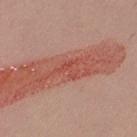Part of a total-body skin-imaging series; this lesion was reviewed on a skin check and was not flagged for biopsy.
The tile uses white-light illumination.
The lesion-visualizer software estimated an automated nevus-likeness rating near 0 out of 100 and lesion-presence confidence of about 60/100.
On the right thigh.
Approximately 3 mm at its widest.
The patient is a female about 30 years old.
A lesion tile, about 15 mm wide, cut from a 3D total-body photograph.